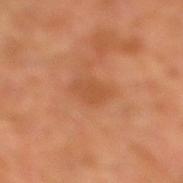<case>
<biopsy_status>not biopsied; imaged during a skin examination</biopsy_status>
<automated_metrics>
  <shape_asymmetry>0.25</shape_asymmetry>
  <border_irregularity_0_10>3.0</border_irregularity_0_10>
  <color_variation_0_10>1.0</color_variation_0_10>
  <peripheral_color_asymmetry>0.5</peripheral_color_asymmetry>
  <nevus_likeness_0_100>5</nevus_likeness_0_100>
  <lesion_detection_confidence_0_100>100</lesion_detection_confidence_0_100>
</automated_metrics>
<site>left leg</site>
<image>
  <source>total-body photography crop</source>
  <field_of_view_mm>15</field_of_view_mm>
</image>
<lesion_size>
  <long_diameter_mm_approx>3.5</long_diameter_mm_approx>
</lesion_size>
<lighting>cross-polarized</lighting>
<patient>
  <sex>male</sex>
  <age_approx>30</age_approx>
</patient>
</case>